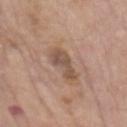Notes:
– notes: catalogued during a skin exam; not biopsied
– lighting: white-light illumination
– lesion size: about 5 mm
– location: the abdomen
– TBP lesion metrics: a footprint of about 7.5 mm² and an eccentricity of roughly 0.95; a mean CIELAB color near L≈52 a*≈17 b*≈27, a lesion–skin lightness drop of about 10, and a normalized border contrast of about 7; a within-lesion color-variation index near 2.5/10 and a peripheral color-asymmetry measure near 0.5; a lesion-detection confidence of about 100/100
– subject: male, roughly 75 years of age
– image source: 15 mm crop, total-body photography